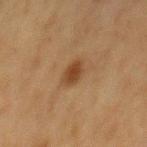biopsy status = no biopsy performed (imaged during a skin exam)
image source = total-body-photography crop, ~15 mm field of view
site = the back
image-analysis metrics = an area of roughly 5 mm² and an eccentricity of roughly 0.7; a mean CIELAB color near L≈35 a*≈17 b*≈29 and a lesion–skin lightness drop of about 8
lighting = cross-polarized
patient = male, aged around 75
lesion size = ~3 mm (longest diameter)